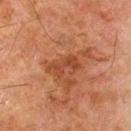{
  "biopsy_status": "not biopsied; imaged during a skin examination",
  "patient": {
    "sex": "male",
    "age_approx": 80
  },
  "site": "left thigh",
  "lighting": "cross-polarized",
  "image": {
    "source": "total-body photography crop",
    "field_of_view_mm": 15
  },
  "automated_metrics": {
    "lesion_detection_confidence_0_100": 100
  }
}